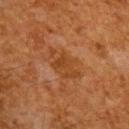{"biopsy_status": "not biopsied; imaged during a skin examination", "image": {"source": "total-body photography crop", "field_of_view_mm": 15}, "patient": {"sex": "male", "age_approx": 65}, "site": "upper back"}